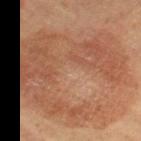* follow-up: imaged on a skin check; not biopsied
* subject: female, roughly 70 years of age
* diameter: ≈12 mm
* acquisition: 15 mm crop, total-body photography
* location: the right thigh
* illumination: cross-polarized